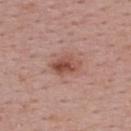Automated tile analysis of the lesion measured a lesion color around L≈50 a*≈24 b*≈27 in CIELAB and about 11 CIELAB-L* units darker than the surrounding skin. The software also gave internal color variation of about 5.5 on a 0–10 scale and a peripheral color-asymmetry measure near 2.5. A region of skin cropped from a whole-body photographic capture, roughly 15 mm wide. Imaged with white-light lighting. A female patient approximately 55 years of age. From the upper back.